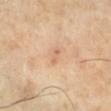Findings:
– workup: no biopsy performed (imaged during a skin exam)
– image: 15 mm crop, total-body photography
– anatomic site: the leg
– patient: female, approximately 70 years of age